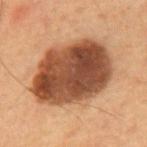{
  "biopsy_status": "not biopsied; imaged during a skin examination",
  "image": {
    "source": "total-body photography crop",
    "field_of_view_mm": 15
  },
  "lighting": "cross-polarized",
  "patient": {
    "sex": "male",
    "age_approx": 60
  },
  "site": "chest"
}